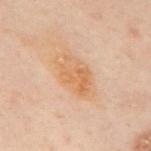{
  "biopsy_status": "not biopsied; imaged during a skin examination",
  "patient": {
    "sex": "male",
    "age_approx": 50
  },
  "site": "mid back",
  "image": {
    "source": "total-body photography crop",
    "field_of_view_mm": 15
  },
  "automated_metrics": {
    "area_mm2_approx": 14.0,
    "eccentricity": 0.8,
    "shape_asymmetry": 0.15,
    "cielab_L": 54,
    "cielab_a": 17,
    "cielab_b": 32,
    "vs_skin_darker_L": 6.0,
    "vs_skin_contrast_norm": 6.5,
    "border_irregularity_0_10": 2.0,
    "peripheral_color_asymmetry": 1.0
  },
  "lesion_size": {
    "long_diameter_mm_approx": 5.5
  },
  "lighting": "cross-polarized"
}